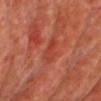Q: Was this lesion biopsied?
A: no biopsy performed (imaged during a skin exam)
Q: What kind of image is this?
A: 15 mm crop, total-body photography
Q: What is the anatomic site?
A: the chest
Q: What are the patient's age and sex?
A: male, approximately 60 years of age